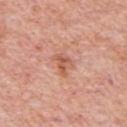workup: total-body-photography surveillance lesion; no biopsy | anatomic site: the chest | tile lighting: white-light illumination | subject: male, aged 78–82 | TBP lesion metrics: a mean CIELAB color near L≈58 a*≈26 b*≈32 and a normalized lesion–skin contrast near 7; internal color variation of about 4.5 on a 0–10 scale and radial color variation of about 2; an automated nevus-likeness rating near 15 out of 100 and a detector confidence of about 100 out of 100 that the crop contains a lesion | lesion size: about 3 mm | acquisition: 15 mm crop, total-body photography.